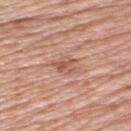Clinical summary:
A close-up tile cropped from a whole-body skin photograph, about 15 mm across. On the upper back. A female subject about 55 years old.